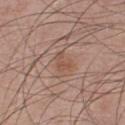Assessment: No biopsy was performed on this lesion — it was imaged during a full skin examination and was not determined to be concerning. Image and clinical context: A male subject, aged around 25. The recorded lesion diameter is about 2.5 mm. Captured under white-light illumination. On the chest. A 15 mm crop from a total-body photograph taken for skin-cancer surveillance.